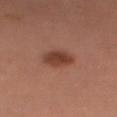The lesion was tiled from a total-body skin photograph and was not biopsied. The tile uses cross-polarized illumination. The subject is a female in their mid-50s. Automated tile analysis of the lesion measured a lesion area of about 8 mm², an eccentricity of roughly 0.8, and a symmetry-axis asymmetry near 0.2. The software also gave a classifier nevus-likeness of about 100/100 and a lesion-detection confidence of about 100/100. Longest diameter approximately 4 mm. On the head or neck. Cropped from a total-body skin-imaging series; the visible field is about 15 mm.